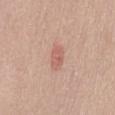<tbp_lesion>
  <biopsy_status>not biopsied; imaged during a skin examination</biopsy_status>
  <image>
    <source>total-body photography crop</source>
    <field_of_view_mm>15</field_of_view_mm>
  </image>
  <patient>
    <sex>male</sex>
    <age_approx>60</age_approx>
  </patient>
  <site>abdomen</site>
</tbp_lesion>